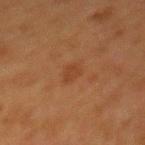Recorded during total-body skin imaging; not selected for excision or biopsy. From the mid back. An algorithmic analysis of the crop reported border irregularity of about 2.5 on a 0–10 scale. The software also gave an automated nevus-likeness rating near 10 out of 100. A 15 mm close-up extracted from a 3D total-body photography capture. The subject is a female aged approximately 50.